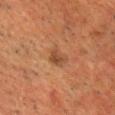Impression: The lesion was photographed on a routine skin check and not biopsied; there is no pathology result. Image and clinical context: A lesion tile, about 15 mm wide, cut from a 3D total-body photograph. A male subject aged around 60. From the front of the torso. The lesion's longest dimension is about 2.5 mm. Captured under cross-polarized illumination.